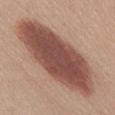{
  "biopsy_status": "not biopsied; imaged during a skin examination",
  "lighting": "white-light",
  "site": "abdomen",
  "image": {
    "source": "total-body photography crop",
    "field_of_view_mm": 15
  },
  "automated_metrics": {
    "eccentricity": 0.9,
    "shape_asymmetry": 0.1,
    "cielab_L": 49,
    "cielab_a": 21,
    "cielab_b": 25,
    "vs_skin_contrast_norm": 11.5
  },
  "lesion_size": {
    "long_diameter_mm_approx": 13.0
  },
  "patient": {
    "sex": "female",
    "age_approx": 55
  }
}